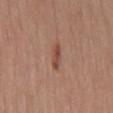The recorded lesion diameter is about 3 mm. This is a white-light tile. A female subject, aged 53 to 57. Automated image analysis of the tile measured an average lesion color of about L≈47 a*≈23 b*≈28 (CIELAB), about 10 CIELAB-L* units darker than the surrounding skin, and a normalized lesion–skin contrast near 7.5. It also reported lesion-presence confidence of about 100/100. The lesion is located on the mid back. This image is a 15 mm lesion crop taken from a total-body photograph.